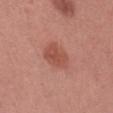Measured at roughly 4 mm in maximum diameter.
On the head or neck.
A roughly 15 mm field-of-view crop from a total-body skin photograph.
This is a white-light tile.
An algorithmic analysis of the crop reported a lesion area of about 8.5 mm², an outline eccentricity of about 0.7 (0 = round, 1 = elongated), and a symmetry-axis asymmetry near 0.15. And it measured an average lesion color of about L≈51 a*≈27 b*≈29 (CIELAB) and a normalized lesion–skin contrast near 6.5. The analysis additionally found an automated nevus-likeness rating near 95 out of 100.
The subject is a female aged 23–27.